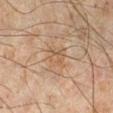acquisition — ~15 mm tile from a whole-body skin photo | subject — male, aged approximately 45 | body site — the leg | lighting — cross-polarized.